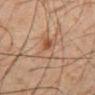No biopsy was performed on this lesion — it was imaged during a full skin examination and was not determined to be concerning.
The lesion-visualizer software estimated a color-variation rating of about 9/10 and radial color variation of about 3.
This image is a 15 mm lesion crop taken from a total-body photograph.
Located on the abdomen.
Approximately 4 mm at its widest.
Imaged with cross-polarized lighting.
A male patient, aged 58 to 62.